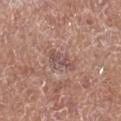Assessment: The lesion was photographed on a routine skin check and not biopsied; there is no pathology result. Image and clinical context: A male patient approximately 80 years of age. Captured under white-light illumination. A lesion tile, about 15 mm wide, cut from a 3D total-body photograph. The lesion is on the leg. The lesion-visualizer software estimated an area of roughly 6 mm², a shape eccentricity near 0.9, and a shape-asymmetry score of about 0.3 (0 = symmetric). And it measured a border-irregularity index near 3.5/10 and a peripheral color-asymmetry measure near 0.5. The analysis additionally found lesion-presence confidence of about 95/100. Measured at roughly 4 mm in maximum diameter.